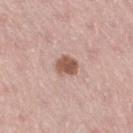The lesion was tiled from a total-body skin photograph and was not biopsied.
A 15 mm close-up extracted from a 3D total-body photography capture.
Measured at roughly 3 mm in maximum diameter.
From the leg.
The subject is a male aged 68 to 72.